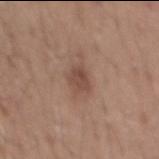Captured during whole-body skin photography for melanoma surveillance; the lesion was not biopsied.
Approximately 2.5 mm at its widest.
Automated tile analysis of the lesion measured a shape eccentricity near 0.65. The analysis additionally found a lesion color around L≈47 a*≈19 b*≈25 in CIELAB and a normalized lesion–skin contrast near 7.
A male subject, aged approximately 55.
The lesion is located on the mid back.
A 15 mm close-up tile from a total-body photography series done for melanoma screening.